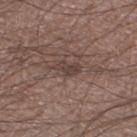workup: no biopsy performed (imaged during a skin exam); lesion size: ≈2.5 mm; site: the right thigh; subject: male, roughly 65 years of age; image: ~15 mm crop, total-body skin-cancer survey; tile lighting: white-light illumination.